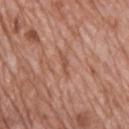Case summary:
- workup — imaged on a skin check; not biopsied
- imaging modality — total-body-photography crop, ~15 mm field of view
- subject — male, about 70 years old
- site — the upper back